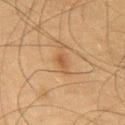Findings:
– biopsy status — total-body-photography surveillance lesion; no biopsy
– tile lighting — cross-polarized illumination
– lesion diameter — ≈2.5 mm
– subject — male, about 75 years old
– automated lesion analysis — a footprint of about 2.5 mm² and a shape eccentricity near 0.9; a mean CIELAB color near L≈43 a*≈18 b*≈32, about 7 CIELAB-L* units darker than the surrounding skin, and a lesion-to-skin contrast of about 6 (normalized; higher = more distinct); a border-irregularity index near 3.5/10
– site — the chest
– image source — ~15 mm crop, total-body skin-cancer survey